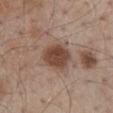Part of a total-body skin-imaging series; this lesion was reviewed on a skin check and was not flagged for biopsy.
A male subject, roughly 55 years of age.
From the mid back.
A 15 mm crop from a total-body photograph taken for skin-cancer surveillance.